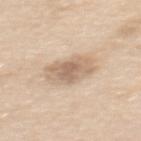notes = total-body-photography surveillance lesion; no biopsy
body site = the mid back
subject = female, in their mid-50s
image = ~15 mm crop, total-body skin-cancer survey